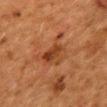notes = catalogued during a skin exam; not biopsied
lighting = cross-polarized illumination
image-analysis metrics = a classifier nevus-likeness of about 5/100 and a lesion-detection confidence of about 100/100
location = the mid back
diameter = ≈5 mm
image source = ~15 mm tile from a whole-body skin photo
subject = female, approximately 50 years of age